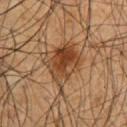Impression:
Recorded during total-body skin imaging; not selected for excision or biopsy.
Context:
A region of skin cropped from a whole-body photographic capture, roughly 15 mm wide. From the chest. The patient is a male aged 63–67.